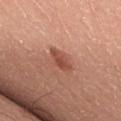  biopsy_status: not biopsied; imaged during a skin examination
  lesion_size:
    long_diameter_mm_approx: 3.0
  site: upper back
  lighting: white-light
  automated_metrics:
    vs_skin_darker_L: 11.0
    vs_skin_contrast_norm: 7.5
    border_irregularity_0_10: 2.5
    color_variation_0_10: 2.5
    peripheral_color_asymmetry: 1.0
    nevus_likeness_0_100: 90
    lesion_detection_confidence_0_100: 100
  patient:
    sex: male
    age_approx: 45
  image:
    source: total-body photography crop
    field_of_view_mm: 15